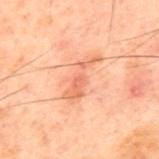Captured during whole-body skin photography for melanoma surveillance; the lesion was not biopsied. A male patient aged 58 to 62. Automated image analysis of the tile measured an outline eccentricity of about 0.95 (0 = round, 1 = elongated) and two-axis asymmetry of about 0.45. The analysis additionally found roughly 7 lightness units darker than nearby skin. The analysis additionally found a color-variation rating of about 2/10 and peripheral color asymmetry of about 0.5. The software also gave an automated nevus-likeness rating near 5 out of 100 and a detector confidence of about 100 out of 100 that the crop contains a lesion. A lesion tile, about 15 mm wide, cut from a 3D total-body photograph. The lesion is on the upper back. The tile uses cross-polarized illumination. Measured at roughly 5.5 mm in maximum diameter.